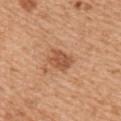Clinical impression:
No biopsy was performed on this lesion — it was imaged during a full skin examination and was not determined to be concerning.
Image and clinical context:
Longest diameter approximately 3 mm. The tile uses white-light illumination. An algorithmic analysis of the crop reported an automated nevus-likeness rating near 60 out of 100 and lesion-presence confidence of about 100/100. From the right upper arm. A region of skin cropped from a whole-body photographic capture, roughly 15 mm wide. A male patient roughly 55 years of age.